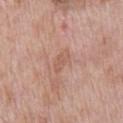Q: Was this lesion biopsied?
A: catalogued during a skin exam; not biopsied
Q: What lighting was used for the tile?
A: white-light illumination
Q: Who is the patient?
A: male, aged 68–72
Q: What is the lesion's diameter?
A: about 3 mm
Q: What is the imaging modality?
A: total-body-photography crop, ~15 mm field of view
Q: What is the anatomic site?
A: the chest